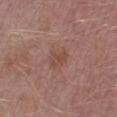Q: Where on the body is the lesion?
A: the right lower leg
Q: What are the patient's age and sex?
A: male, in their mid-50s
Q: What is the lesion's diameter?
A: ≈2.5 mm
Q: How was the tile lit?
A: white-light
Q: What is the imaging modality?
A: 15 mm crop, total-body photography
Q: Automated lesion metrics?
A: a lesion color around L≈47 a*≈20 b*≈25 in CIELAB and a normalized lesion–skin contrast near 5; internal color variation of about 2 on a 0–10 scale and peripheral color asymmetry of about 1